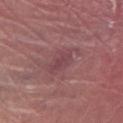Imaged during a routine full-body skin examination; the lesion was not biopsied and no histopathology is available.
A male patient aged around 70.
The total-body-photography lesion software estimated an average lesion color of about L≈43 a*≈26 b*≈16 (CIELAB) and a lesion–skin lightness drop of about 7. The software also gave an automated nevus-likeness rating near 0 out of 100 and a detector confidence of about 80 out of 100 that the crop contains a lesion.
The recorded lesion diameter is about 3 mm.
From the left lower leg.
A lesion tile, about 15 mm wide, cut from a 3D total-body photograph.
Imaged with white-light lighting.